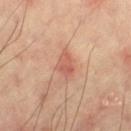The lesion was tiled from a total-body skin photograph and was not biopsied. The tile uses cross-polarized illumination. Approximately 3.5 mm at its widest. A 15 mm crop from a total-body photograph taken for skin-cancer surveillance. The lesion is located on the left thigh. The total-body-photography lesion software estimated a lesion area of about 5.5 mm² and a symmetry-axis asymmetry near 0.2. It also reported a border-irregularity index near 2.5/10, a color-variation rating of about 3.5/10, and radial color variation of about 1.5. The analysis additionally found a detector confidence of about 100 out of 100 that the crop contains a lesion.